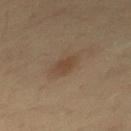Located on the mid back.
Captured under cross-polarized illumination.
A male patient aged around 35.
A lesion tile, about 15 mm wide, cut from a 3D total-body photograph.